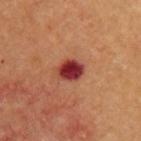Recorded during total-body skin imaging; not selected for excision or biopsy. A roughly 15 mm field-of-view crop from a total-body skin photograph. The lesion's longest dimension is about 3 mm. The patient is a male in their mid-60s. Automated tile analysis of the lesion measured an area of roughly 6 mm² and a shape eccentricity near 0.65. And it measured a mean CIELAB color near L≈33 a*≈33 b*≈25 and a normalized lesion–skin contrast near 14.5. And it measured a border-irregularity index near 2/10 and a within-lesion color-variation index near 5.5/10. The analysis additionally found an automated nevus-likeness rating near 0 out of 100 and a lesion-detection confidence of about 100/100. Located on the right upper arm. The tile uses cross-polarized illumination.